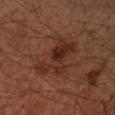Assessment:
The lesion was tiled from a total-body skin photograph and was not biopsied.
Context:
A close-up tile cropped from a whole-body skin photograph, about 15 mm across. A male subject approximately 65 years of age. Located on the left arm.